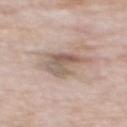acquisition=~15 mm tile from a whole-body skin photo | lighting=white-light | site=the mid back | subject=male, in their mid- to late 70s | image-analysis metrics=a lesion area of about 11 mm² and two-axis asymmetry of about 0.4; roughly 11 lightness units darker than nearby skin and a normalized border contrast of about 7; a within-lesion color-variation index near 7.5/10 and peripheral color asymmetry of about 3 | lesion size=≈4.5 mm.